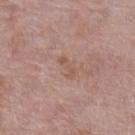{
  "biopsy_status": "not biopsied; imaged during a skin examination",
  "lesion_size": {
    "long_diameter_mm_approx": 3.0
  },
  "lighting": "white-light",
  "site": "leg",
  "patient": {
    "sex": "female",
    "age_approx": 70
  },
  "image": {
    "source": "total-body photography crop",
    "field_of_view_mm": 15
  },
  "automated_metrics": {
    "area_mm2_approx": 2.5,
    "eccentricity": 0.95,
    "shape_asymmetry": 0.25,
    "cielab_L": 54,
    "cielab_a": 20,
    "cielab_b": 26,
    "vs_skin_darker_L": 6.0,
    "vs_skin_contrast_norm": 5.0,
    "border_irregularity_0_10": 4.0,
    "color_variation_0_10": 0.0,
    "peripheral_color_asymmetry": 0.0,
    "nevus_likeness_0_100": 0,
    "lesion_detection_confidence_0_100": 100
  }
}